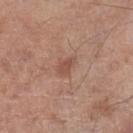subject = male, approximately 70 years of age; image source = ~15 mm crop, total-body skin-cancer survey; location = the leg.